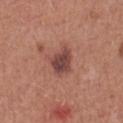Imaged during a routine full-body skin examination; the lesion was not biopsied and no histopathology is available. The tile uses white-light illumination. A female patient, about 25 years old. About 3 mm across. From the chest. A region of skin cropped from a whole-body photographic capture, roughly 15 mm wide.